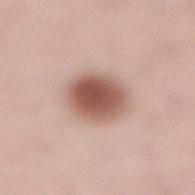Findings:
• biopsy status: catalogued during a skin exam; not biopsied
• image: total-body-photography crop, ~15 mm field of view
• lighting: white-light illumination
• lesion diameter: ≈4.5 mm
• automated lesion analysis: a normalized border contrast of about 10.5; border irregularity of about 0.5 on a 0–10 scale
• subject: female, aged approximately 50
• location: the lower back